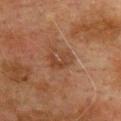<record>
  <biopsy_status>not biopsied; imaged during a skin examination</biopsy_status>
</record>